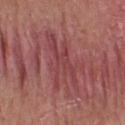biopsy status — imaged on a skin check; not biopsied
tile lighting — white-light
image source — ~15 mm crop, total-body skin-cancer survey
body site — the upper back
diameter — ≈6.5 mm
subject — male, aged around 40